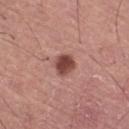Recorded during total-body skin imaging; not selected for excision or biopsy.
The patient is a male roughly 65 years of age.
From the right thigh.
A close-up tile cropped from a whole-body skin photograph, about 15 mm across.
Longest diameter approximately 2.5 mm.